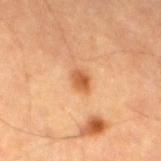Q: Is there a histopathology result?
A: imaged on a skin check; not biopsied
Q: Lesion location?
A: the left thigh
Q: What did automated image analysis measure?
A: a footprint of about 4 mm² and a shape eccentricity near 0.7; a lesion color around L≈51 a*≈24 b*≈37 in CIELAB and a lesion–skin lightness drop of about 11; a border-irregularity index near 1.5/10, a within-lesion color-variation index near 3/10, and a peripheral color-asymmetry measure near 1; a classifier nevus-likeness of about 80/100 and a lesion-detection confidence of about 100/100
Q: How large is the lesion?
A: about 2.5 mm
Q: What is the imaging modality?
A: total-body-photography crop, ~15 mm field of view
Q: Patient demographics?
A: male, aged around 85 or older
Q: How was the tile lit?
A: cross-polarized